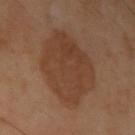The lesion was tiled from a total-body skin photograph and was not biopsied.